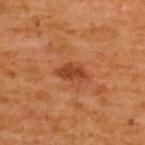Clinical impression: No biopsy was performed on this lesion — it was imaged during a full skin examination and was not determined to be concerning. Image and clinical context: Imaged with cross-polarized lighting. Longest diameter approximately 3.5 mm. The lesion is located on the back. The patient is a male aged 63–67. Automated image analysis of the tile measured a classifier nevus-likeness of about 30/100 and lesion-presence confidence of about 100/100. A 15 mm crop from a total-body photograph taken for skin-cancer surveillance.